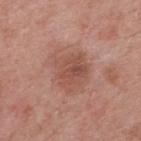| key | value |
|---|---|
| biopsy status | catalogued during a skin exam; not biopsied |
| lighting | white-light |
| image | ~15 mm crop, total-body skin-cancer survey |
| patient | female, roughly 60 years of age |
| TBP lesion metrics | a footprint of about 17 mm², an outline eccentricity of about 0.55 (0 = round, 1 = elongated), and two-axis asymmetry of about 0.3; a mean CIELAB color near L≈52 a*≈23 b*≈27 and a normalized lesion–skin contrast near 6; a classifier nevus-likeness of about 0/100 |
| diameter | ≈5.5 mm |
| anatomic site | the upper back |